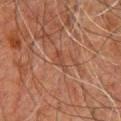This lesion was catalogued during total-body skin photography and was not selected for biopsy.
Measured at roughly 3 mm in maximum diameter.
From the chest.
The lesion-visualizer software estimated an eccentricity of roughly 0.9 and a symmetry-axis asymmetry near 0.4. And it measured an average lesion color of about L≈35 a*≈19 b*≈25 (CIELAB) and roughly 5 lightness units darker than nearby skin. And it measured a border-irregularity index near 4.5/10.
This image is a 15 mm lesion crop taken from a total-body photograph.
A male patient, aged 58–62.